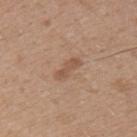notes — imaged on a skin check; not biopsied | illumination — white-light | anatomic site — the upper back | automated lesion analysis — a lesion area of about 3.5 mm², an outline eccentricity of about 0.95 (0 = round, 1 = elongated), and a shape-asymmetry score of about 0.25 (0 = symmetric); a mean CIELAB color near L≈53 a*≈19 b*≈30, roughly 8 lightness units darker than nearby skin, and a lesion-to-skin contrast of about 6 (normalized; higher = more distinct) | acquisition — ~15 mm crop, total-body skin-cancer survey | subject — male, in their 50s | lesion size — ≈3.5 mm.